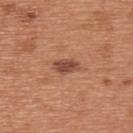notes: no biopsy performed (imaged during a skin exam)